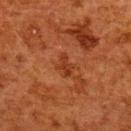  biopsy_status: not biopsied; imaged during a skin examination
  image:
    source: total-body photography crop
    field_of_view_mm: 15
  site: upper back
  patient:
    sex: female
    age_approx: 50
  lighting: cross-polarized
  automated_metrics:
    area_mm2_approx: 3.0
    shape_asymmetry: 0.4
  lesion_size:
    long_diameter_mm_approx: 2.5